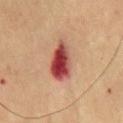Impression:
Captured during whole-body skin photography for melanoma surveillance; the lesion was not biopsied.
Clinical summary:
The lesion-visualizer software estimated an area of roughly 11 mm², a shape eccentricity near 0.9, and two-axis asymmetry of about 0.25. The analysis additionally found a normalized border contrast of about 13. Captured under cross-polarized illumination. A lesion tile, about 15 mm wide, cut from a 3D total-body photograph. A male patient approximately 70 years of age. Located on the chest. Measured at roughly 5.5 mm in maximum diameter.